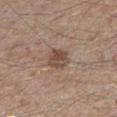The lesion was photographed on a routine skin check and not biopsied; there is no pathology result.
On the left lower leg.
The patient is a male in their mid- to late 60s.
A 15 mm close-up extracted from a 3D total-body photography capture.
Captured under white-light illumination.